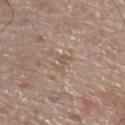Impression:
The lesion was photographed on a routine skin check and not biopsied; there is no pathology result.
Image and clinical context:
A male subject aged approximately 70. The tile uses white-light illumination. The lesion is on the left lower leg. Measured at roughly 2.5 mm in maximum diameter. The total-body-photography lesion software estimated a footprint of about 2.5 mm². And it measured an average lesion color of about L≈54 a*≈17 b*≈26 (CIELAB) and about 6 CIELAB-L* units darker than the surrounding skin. A lesion tile, about 15 mm wide, cut from a 3D total-body photograph.